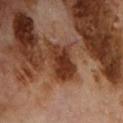Impression: Captured during whole-body skin photography for melanoma surveillance; the lesion was not biopsied. Background: The lesion's longest dimension is about 5 mm. The tile uses cross-polarized illumination. The lesion is located on the chest. Cropped from a whole-body photographic skin survey; the tile spans about 15 mm. A male subject aged around 70. The lesion-visualizer software estimated an area of roughly 11 mm², an eccentricity of roughly 0.8, and a symmetry-axis asymmetry near 0.35. The software also gave a mean CIELAB color near L≈34 a*≈23 b*≈30, roughly 12 lightness units darker than nearby skin, and a normalized border contrast of about 10. The software also gave border irregularity of about 4 on a 0–10 scale and radial color variation of about 1.5. The software also gave a classifier nevus-likeness of about 20/100 and a detector confidence of about 95 out of 100 that the crop contains a lesion.